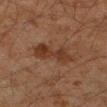The lesion was tiled from a total-body skin photograph and was not biopsied.
A close-up tile cropped from a whole-body skin photograph, about 15 mm across.
Captured under cross-polarized illumination.
The recorded lesion diameter is about 5.5 mm.
The subject is a male aged 43 to 47.
Located on the left forearm.